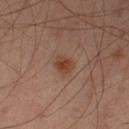Recorded during total-body skin imaging; not selected for excision or biopsy.